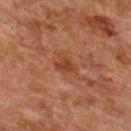A region of skin cropped from a whole-body photographic capture, roughly 15 mm wide. Located on the upper back. A female subject, aged around 60.